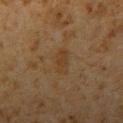No biopsy was performed on this lesion — it was imaged during a full skin examination and was not determined to be concerning.
On the arm.
A male patient, approximately 60 years of age.
The lesion-visualizer software estimated a lesion color around L≈31 a*≈14 b*≈27 in CIELAB and a normalized border contrast of about 5. It also reported radial color variation of about 0.5. And it measured a lesion-detection confidence of about 100/100.
A close-up tile cropped from a whole-body skin photograph, about 15 mm across.
This is a cross-polarized tile.